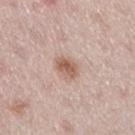  biopsy_status: not biopsied; imaged during a skin examination